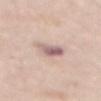No biopsy was performed on this lesion — it was imaged during a full skin examination and was not determined to be concerning. The tile uses white-light illumination. The lesion is located on the mid back. Approximately 4 mm at its widest. A 15 mm close-up extracted from a 3D total-body photography capture. A female subject, in their mid-60s. The lesion-visualizer software estimated a mean CIELAB color near L≈63 a*≈18 b*≈20 and a normalized border contrast of about 8. It also reported a nevus-likeness score of about 0/100 and lesion-presence confidence of about 95/100.